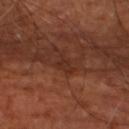<tbp_lesion>
  <biopsy_status>not biopsied; imaged during a skin examination</biopsy_status>
  <lesion_size>
    <long_diameter_mm_approx>3.0</long_diameter_mm_approx>
  </lesion_size>
  <image>
    <source>total-body photography crop</source>
    <field_of_view_mm>15</field_of_view_mm>
  </image>
  <site>left lower leg</site>
  <lighting>cross-polarized</lighting>
  <automated_metrics>
    <area_mm2_approx>3.0</area_mm2_approx>
    <eccentricity>0.85</eccentricity>
    <shape_asymmetry>0.5</shape_asymmetry>
    <border_irregularity_0_10>5.5</border_irregularity_0_10>
    <color_variation_0_10>0.5</color_variation_0_10>
    <peripheral_color_asymmetry>0.5</peripheral_color_asymmetry>
  </automated_metrics>
  <patient>
    <age_approx>65</age_approx>
  </patient>
</tbp_lesion>